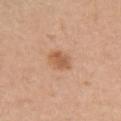The lesion was tiled from a total-body skin photograph and was not biopsied. Cropped from a total-body skin-imaging series; the visible field is about 15 mm. A female patient, in their mid- to late 40s. From the chest. Measured at roughly 3 mm in maximum diameter. Automated tile analysis of the lesion measured an area of roughly 5 mm², a shape eccentricity near 0.7, and a shape-asymmetry score of about 0.2 (0 = symmetric). The software also gave a color-variation rating of about 2/10. It also reported an automated nevus-likeness rating near 80 out of 100 and lesion-presence confidence of about 100/100.